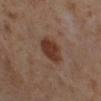<case>
  <biopsy_status>not biopsied; imaged during a skin examination</biopsy_status>
  <lighting>cross-polarized</lighting>
  <patient>
    <sex>female</sex>
    <age_approx>50</age_approx>
  </patient>
  <lesion_size>
    <long_diameter_mm_approx>4.0</long_diameter_mm_approx>
  </lesion_size>
  <site>right lower leg</site>
  <image>
    <source>total-body photography crop</source>
    <field_of_view_mm>15</field_of_view_mm>
  </image>
</case>